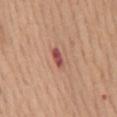The lesion was tiled from a total-body skin photograph and was not biopsied. A 15 mm crop from a total-body photograph taken for skin-cancer surveillance. Located on the mid back. Measured at roughly 2.5 mm in maximum diameter. A female subject in their mid-60s. Automated tile analysis of the lesion measured an automated nevus-likeness rating near 0 out of 100. Imaged with white-light lighting.